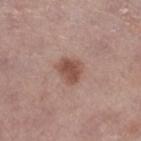{
  "biopsy_status": "not biopsied; imaged during a skin examination",
  "patient": {
    "sex": "female",
    "age_approx": 65
  },
  "site": "right lower leg",
  "image": {
    "source": "total-body photography crop",
    "field_of_view_mm": 15
  }
}